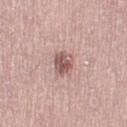This lesion was catalogued during total-body skin photography and was not selected for biopsy.
A close-up tile cropped from a whole-body skin photograph, about 15 mm across.
Automated image analysis of the tile measured an average lesion color of about L≈56 a*≈21 b*≈22 (CIELAB) and roughly 13 lightness units darker than nearby skin. And it measured a lesion-detection confidence of about 100/100.
Imaged with white-light lighting.
On the right thigh.
Approximately 3 mm at its widest.
A female patient, aged 63–67.